A female subject roughly 35 years of age; on the chest; a lesion tile, about 15 mm wide, cut from a 3D total-body photograph:
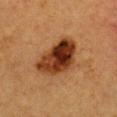Longest diameter approximately 6 mm. This is a cross-polarized tile. On excision, pathology confirmed a melanoma in situ, classified as a skin cancer.